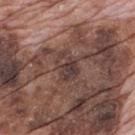  automated_metrics:
    area_mm2_approx: 6.5
    eccentricity: 0.7
    shape_asymmetry: 0.25
  patient:
    sex: male
    age_approx: 70
  lesion_size:
    long_diameter_mm_approx: 3.5
  lighting: white-light
  image:
    source: total-body photography crop
    field_of_view_mm: 15
  site: mid back